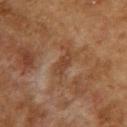Context:
A 15 mm close-up extracted from a 3D total-body photography capture. The recorded lesion diameter is about 3 mm. The total-body-photography lesion software estimated an average lesion color of about L≈36 a*≈18 b*≈28 (CIELAB), about 7 CIELAB-L* units darker than the surrounding skin, and a normalized border contrast of about 6. It also reported border irregularity of about 3.5 on a 0–10 scale and a color-variation rating of about 1/10. The lesion is on the upper back. A female patient roughly 60 years of age. Imaged with cross-polarized lighting.